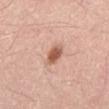Captured during whole-body skin photography for melanoma surveillance; the lesion was not biopsied. Longest diameter approximately 3 mm. Automated image analysis of the tile measured a footprint of about 4.5 mm², a shape eccentricity near 0.7, and a symmetry-axis asymmetry near 0.2. The analysis additionally found a mean CIELAB color near L≈58 a*≈24 b*≈31, a lesion–skin lightness drop of about 14, and a normalized border contrast of about 9.5. And it measured a border-irregularity index near 1.5/10, a color-variation rating of about 3/10, and a peripheral color-asymmetry measure near 1. From the mid back. Captured under white-light illumination. A 15 mm close-up extracted from a 3D total-body photography capture. A male subject about 60 years old.